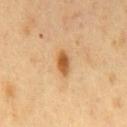Findings:
• biopsy status: catalogued during a skin exam; not biopsied
• site: the chest
• illumination: cross-polarized illumination
• image source: ~15 mm crop, total-body skin-cancer survey
• patient: male, aged 48–52
• lesion diameter: ≈3 mm